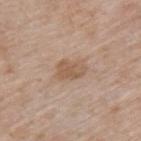notes: imaged on a skin check; not biopsied | body site: the upper back | image source: ~15 mm crop, total-body skin-cancer survey | patient: male, roughly 60 years of age.